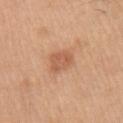biopsy_status: not biopsied; imaged during a skin examination
image:
  source: total-body photography crop
  field_of_view_mm: 15
patient:
  sex: female
  age_approx: 55
site: chest
lighting: white-light
lesion_size:
  long_diameter_mm_approx: 3.5